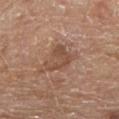follow-up — catalogued during a skin exam; not biopsied.